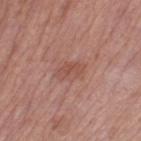Notes:
* notes — catalogued during a skin exam; not biopsied
* location — the left thigh
* tile lighting — white-light
* image — 15 mm crop, total-body photography
* diameter — ~3 mm (longest diameter)
* subject — female, aged approximately 55